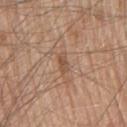Imaged during a routine full-body skin examination; the lesion was not biopsied and no histopathology is available. The tile uses white-light illumination. On the front of the torso. The recorded lesion diameter is about 2.5 mm. This image is a 15 mm lesion crop taken from a total-body photograph. A male patient, aged around 80.